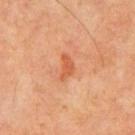biopsy_status: not biopsied; imaged during a skin examination
lighting: cross-polarized
lesion_size:
  long_diameter_mm_approx: 3.0
site: chest
image:
  source: total-body photography crop
  field_of_view_mm: 15
automated_metrics:
  cielab_L: 55
  cielab_a: 29
  cielab_b: 39
  nevus_likeness_0_100: 0
  lesion_detection_confidence_0_100: 100
patient:
  sex: male
  age_approx: 65